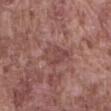Impression:
Recorded during total-body skin imaging; not selected for excision or biopsy.
Context:
Approximately 4 mm at its widest. A male subject roughly 75 years of age. A 15 mm close-up tile from a total-body photography series done for melanoma screening. On the abdomen. This is a white-light tile.